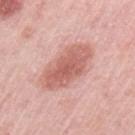Clinical impression: The lesion was photographed on a routine skin check and not biopsied; there is no pathology result. Acquisition and patient details: The subject is a female in their mid-60s. On the left upper arm. The tile uses white-light illumination. About 7 mm across. A region of skin cropped from a whole-body photographic capture, roughly 15 mm wide.